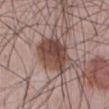Findings:
– follow-up · catalogued during a skin exam; not biopsied
– location · the front of the torso
– imaging modality · ~15 mm tile from a whole-body skin photo
– size · ~5.5 mm (longest diameter)
– patient · male, about 45 years old
– illumination · white-light illumination
– TBP lesion metrics · border irregularity of about 3.5 on a 0–10 scale, internal color variation of about 7 on a 0–10 scale, and peripheral color asymmetry of about 2.5